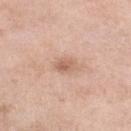Recorded during total-body skin imaging; not selected for excision or biopsy. The lesion is located on the left lower leg. A 15 mm crop from a total-body photograph taken for skin-cancer surveillance. The recorded lesion diameter is about 2.5 mm. The lesion-visualizer software estimated an area of roughly 3.5 mm², an outline eccentricity of about 0.75 (0 = round, 1 = elongated), and two-axis asymmetry of about 0.15. And it measured an average lesion color of about L≈62 a*≈20 b*≈30 (CIELAB), a lesion–skin lightness drop of about 10, and a normalized border contrast of about 6.5. The analysis additionally found a nevus-likeness score of about 5/100 and a detector confidence of about 100 out of 100 that the crop contains a lesion. The tile uses white-light illumination. The subject is a female in their mid-50s.